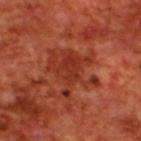notes=imaged on a skin check; not biopsied | lesion diameter=≈6 mm | subject=male, aged approximately 70 | site=the upper back | acquisition=15 mm crop, total-body photography | tile lighting=cross-polarized illumination.